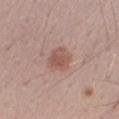Case summary:
– notes — total-body-photography surveillance lesion; no biopsy